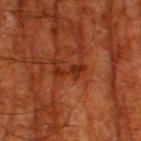Assessment: Part of a total-body skin-imaging series; this lesion was reviewed on a skin check and was not flagged for biopsy. Context: A male patient aged around 80. Cropped from a whole-body photographic skin survey; the tile spans about 15 mm. On the left thigh.